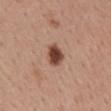Notes:
* follow-up — catalogued during a skin exam; not biopsied
* tile lighting — white-light illumination
* location — the mid back
* image-analysis metrics — an average lesion color of about L≈45 a*≈22 b*≈28 (CIELAB), a lesion–skin lightness drop of about 17, and a lesion-to-skin contrast of about 12 (normalized; higher = more distinct); a within-lesion color-variation index near 3.5/10 and radial color variation of about 1
* subject — female, about 55 years old
* size — ≈2.5 mm
* acquisition — total-body-photography crop, ~15 mm field of view